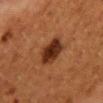No biopsy was performed on this lesion — it was imaged during a full skin examination and was not determined to be concerning. From the back. Longest diameter approximately 4 mm. The patient is a female aged 48 to 52. Automated image analysis of the tile measured an outline eccentricity of about 0.7 (0 = round, 1 = elongated) and two-axis asymmetry of about 0.2. The software also gave a lesion color around L≈25 a*≈21 b*≈27 in CIELAB, a lesion–skin lightness drop of about 12, and a normalized border contrast of about 12.5. And it measured a border-irregularity index near 2/10, a color-variation rating of about 3.5/10, and a peripheral color-asymmetry measure near 1. This image is a 15 mm lesion crop taken from a total-body photograph. Captured under cross-polarized illumination.